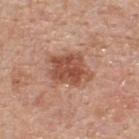follow-up = catalogued during a skin exam; not biopsied
site = the upper back
subject = male, roughly 55 years of age
acquisition = 15 mm crop, total-body photography